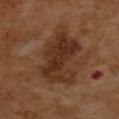No biopsy was performed on this lesion — it was imaged during a full skin examination and was not determined to be concerning. This image is a 15 mm lesion crop taken from a total-body photograph. A female subject, aged 53–57. Imaged with cross-polarized lighting. The recorded lesion diameter is about 7 mm. The total-body-photography lesion software estimated a lesion area of about 24 mm², an eccentricity of roughly 0.8, and a shape-asymmetry score of about 0.35 (0 = symmetric). And it measured roughly 9 lightness units darker than nearby skin and a normalized border contrast of about 9. It also reported a classifier nevus-likeness of about 0/100 and lesion-presence confidence of about 100/100. Located on the upper back.